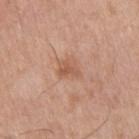{
  "biopsy_status": "not biopsied; imaged during a skin examination"
}